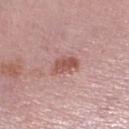Clinical impression:
Imaged during a routine full-body skin examination; the lesion was not biopsied and no histopathology is available.
Clinical summary:
Measured at roughly 3 mm in maximum diameter. Imaged with white-light lighting. The subject is a female about 50 years old. Cropped from a total-body skin-imaging series; the visible field is about 15 mm. The lesion is located on the right lower leg.